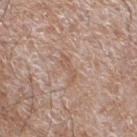Recorded during total-body skin imaging; not selected for excision or biopsy.
The patient is a male aged around 60.
A close-up tile cropped from a whole-body skin photograph, about 15 mm across.
The lesion is located on the left lower leg.